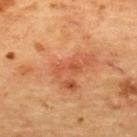| field | value |
|---|---|
| biopsy status | total-body-photography surveillance lesion; no biopsy |
| body site | the upper back |
| image | total-body-photography crop, ~15 mm field of view |
| lesion size | ~3 mm (longest diameter) |
| subject | female, approximately 70 years of age |
| illumination | cross-polarized |